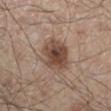follow-up: catalogued during a skin exam; not biopsied
image source: total-body-photography crop, ~15 mm field of view
lighting: white-light
anatomic site: the left lower leg
patient: male, approximately 45 years of age
image-analysis metrics: a footprint of about 12 mm², an outline eccentricity of about 0.35 (0 = round, 1 = elongated), and two-axis asymmetry of about 0.2; about 13 CIELAB-L* units darker than the surrounding skin; border irregularity of about 2 on a 0–10 scale, a color-variation rating of about 5.5/10, and peripheral color asymmetry of about 1.5